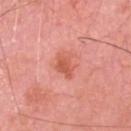Findings:
- biopsy status — catalogued during a skin exam; not biopsied
- automated metrics — a lesion area of about 5.5 mm², an outline eccentricity of about 0.7 (0 = round, 1 = elongated), and two-axis asymmetry of about 0.3; a lesion color around L≈59 a*≈33 b*≈34 in CIELAB and a lesion–skin lightness drop of about 9; a within-lesion color-variation index near 2.5/10 and a peripheral color-asymmetry measure near 0.5
- image — 15 mm crop, total-body photography
- subject — male, approximately 80 years of age
- size — ≈3 mm
- body site — the head or neck
- lighting — white-light